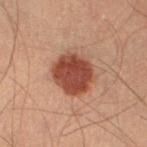Clinical impression:
Recorded during total-body skin imaging; not selected for excision or biopsy.
Acquisition and patient details:
A male subject, roughly 40 years of age. The lesion is on the leg. Automated image analysis of the tile measured a lesion area of about 15 mm², an outline eccentricity of about 0.5 (0 = round, 1 = elongated), and two-axis asymmetry of about 0.15. The software also gave an average lesion color of about L≈40 a*≈25 b*≈28 (CIELAB), about 14 CIELAB-L* units darker than the surrounding skin, and a normalized border contrast of about 11. And it measured a border-irregularity index near 1.5/10, internal color variation of about 3.5 on a 0–10 scale, and a peripheral color-asymmetry measure near 1. The analysis additionally found a classifier nevus-likeness of about 100/100. A roughly 15 mm field-of-view crop from a total-body skin photograph. The recorded lesion diameter is about 4.5 mm.